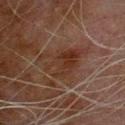Case summary:
- workup · no biopsy performed (imaged during a skin exam)
- image source · total-body-photography crop, ~15 mm field of view
- site · the chest
- subject · male, approximately 80 years of age
- lighting · cross-polarized illumination
- lesion diameter · about 6.5 mm